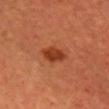  biopsy_status: not biopsied; imaged during a skin examination
  image:
    source: total-body photography crop
    field_of_view_mm: 15
  lighting: cross-polarized
  patient:
    sex: female
    age_approx: 45
  site: front of the torso
  lesion_size:
    long_diameter_mm_approx: 3.5